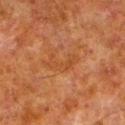The tile uses cross-polarized illumination.
Located on the left lower leg.
A male patient, in their 80s.
The lesion-visualizer software estimated a footprint of about 5 mm² and a shape eccentricity near 0.9. The analysis additionally found a border-irregularity index near 9.5/10 and internal color variation of about 0 on a 0–10 scale.
A close-up tile cropped from a whole-body skin photograph, about 15 mm across.